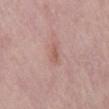location=the back
image source=15 mm crop, total-body photography
patient=male, aged around 55
tile lighting=white-light